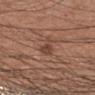Part of a total-body skin-imaging series; this lesion was reviewed on a skin check and was not flagged for biopsy.
The lesion is on the right forearm.
The recorded lesion diameter is about 4 mm.
Imaged with white-light lighting.
This image is a 15 mm lesion crop taken from a total-body photograph.
The patient is a male aged 28–32.
An algorithmic analysis of the crop reported a footprint of about 4.5 mm² and an eccentricity of roughly 0.9. And it measured an average lesion color of about L≈44 a*≈20 b*≈26 (CIELAB) and a lesion-to-skin contrast of about 6.5 (normalized; higher = more distinct). The software also gave border irregularity of about 5 on a 0–10 scale and a within-lesion color-variation index near 2/10.